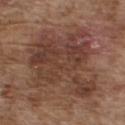<record>
  <biopsy_status>not biopsied; imaged during a skin examination</biopsy_status>
  <site>front of the torso</site>
  <image>
    <source>total-body photography crop</source>
    <field_of_view_mm>15</field_of_view_mm>
  </image>
  <lesion_size>
    <long_diameter_mm_approx>9.0</long_diameter_mm_approx>
  </lesion_size>
  <patient>
    <sex>male</sex>
    <age_approx>75</age_approx>
  </patient>
  <lighting>white-light</lighting>
</record>